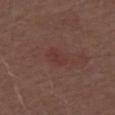Findings:
* workup: no biopsy performed (imaged during a skin exam)
* anatomic site: the right upper arm
* patient: male, aged 68 to 72
* size: ~3 mm (longest diameter)
* tile lighting: white-light illumination
* image: total-body-photography crop, ~15 mm field of view